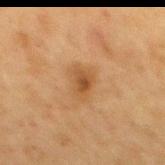Case summary:
- notes · no biopsy performed (imaged during a skin exam)
- automated metrics · an average lesion color of about L≈42 a*≈17 b*≈33 (CIELAB), a lesion–skin lightness drop of about 8, and a normalized border contrast of about 6.5; border irregularity of about 3 on a 0–10 scale, a within-lesion color-variation index near 3.5/10, and peripheral color asymmetry of about 1
- subject · male, roughly 75 years of age
- diameter · about 3.5 mm
- illumination · cross-polarized illumination
- body site · the mid back
- image source · 15 mm crop, total-body photography